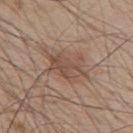A male subject, aged 48 to 52. A close-up tile cropped from a whole-body skin photograph, about 15 mm across. The tile uses white-light illumination. The total-body-photography lesion software estimated an area of roughly 11 mm², an eccentricity of roughly 0.75, and a symmetry-axis asymmetry near 0.35. It also reported a lesion color around L≈49 a*≈18 b*≈25 in CIELAB, roughly 8 lightness units darker than nearby skin, and a normalized border contrast of about 6. It also reported a border-irregularity index near 5/10 and internal color variation of about 3 on a 0–10 scale. The software also gave a classifier nevus-likeness of about 10/100 and a lesion-detection confidence of about 100/100. The lesion is located on the upper back.